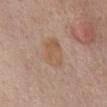Assessment:
The lesion was tiled from a total-body skin photograph and was not biopsied.
Image and clinical context:
The lesion is located on the abdomen. The subject is a female in their mid- to late 60s. Cropped from a whole-body photographic skin survey; the tile spans about 15 mm.